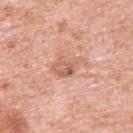| feature | finding |
|---|---|
| TBP lesion metrics | a footprint of about 7.5 mm², an outline eccentricity of about 0.8 (0 = round, 1 = elongated), and a shape-asymmetry score of about 0.3 (0 = symmetric); an average lesion color of about L≈62 a*≈23 b*≈31 (CIELAB), roughly 9 lightness units darker than nearby skin, and a lesion-to-skin contrast of about 6 (normalized; higher = more distinct); a border-irregularity rating of about 4/10 and a peripheral color-asymmetry measure near 2; a classifier nevus-likeness of about 0/100 and a detector confidence of about 100 out of 100 that the crop contains a lesion |
| tile lighting | white-light illumination |
| image | total-body-photography crop, ~15 mm field of view |
| site | the upper back |
| patient | male, aged 78 to 82 |
| diameter | ≈4 mm |